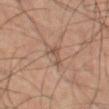The lesion was tiled from a total-body skin photograph and was not biopsied.
A close-up tile cropped from a whole-body skin photograph, about 15 mm across.
On the left thigh.
Approximately 3 mm at its widest.
A male subject, roughly 60 years of age.
Captured under cross-polarized illumination.